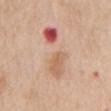  biopsy_status: not biopsied; imaged during a skin examination
  patient:
    sex: female
    age_approx: 65
  automated_metrics:
    cielab_L: 63
    cielab_a: 21
    cielab_b: 30
    vs_skin_darker_L: 8.0
    vs_skin_contrast_norm: 5.5
    nevus_likeness_0_100: 0
    lesion_detection_confidence_0_100: 100
  site: abdomen
  image:
    source: total-body photography crop
    field_of_view_mm: 15
  lighting: white-light
  lesion_size:
    long_diameter_mm_approx: 8.0